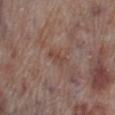notes: total-body-photography surveillance lesion; no biopsy | automated lesion analysis: an outline eccentricity of about 0.9 (0 = round, 1 = elongated) and a shape-asymmetry score of about 0.3 (0 = symmetric); a mean CIELAB color near L≈44 a*≈19 b*≈23 and roughly 7 lightness units darker than nearby skin; a detector confidence of about 100 out of 100 that the crop contains a lesion | location: the right lower leg | patient: male, roughly 70 years of age | illumination: white-light | image source: 15 mm crop, total-body photography | diameter: ~2.5 mm (longest diameter).